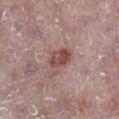follow-up=catalogued during a skin exam; not biopsied
anatomic site=the right lower leg
patient=male, aged around 75
size=about 3.5 mm
acquisition=~15 mm tile from a whole-body skin photo